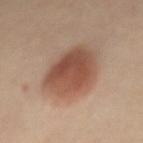Recorded during total-body skin imaging; not selected for excision or biopsy. This is a cross-polarized tile. Automated tile analysis of the lesion measured an automated nevus-likeness rating near 100 out of 100 and lesion-presence confidence of about 100/100. Located on the abdomen. A region of skin cropped from a whole-body photographic capture, roughly 15 mm wide. The patient is a female approximately 45 years of age.